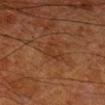Notes:
- follow-up · total-body-photography surveillance lesion; no biopsy
- subject · male, aged 78–82
- body site · the leg
- image · 15 mm crop, total-body photography
- lighting · cross-polarized illumination